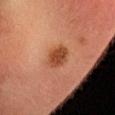This lesion was catalogued during total-body skin photography and was not selected for biopsy. The lesion's longest dimension is about 3 mm. Cropped from a whole-body photographic skin survey; the tile spans about 15 mm. An algorithmic analysis of the crop reported an average lesion color of about L≈42 a*≈25 b*≈33 (CIELAB), roughly 11 lightness units darker than nearby skin, and a normalized lesion–skin contrast near 9. Located on the head or neck. Captured under cross-polarized illumination. A male subject roughly 35 years of age.